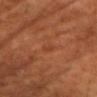Q: Was a biopsy performed?
A: imaged on a skin check; not biopsied
Q: How large is the lesion?
A: ≈2 mm
Q: Patient demographics?
A: male, approximately 60 years of age
Q: What is the imaging modality?
A: ~15 mm crop, total-body skin-cancer survey
Q: How was the tile lit?
A: cross-polarized illumination
Q: Where on the body is the lesion?
A: the chest